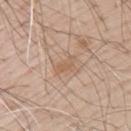Captured during whole-body skin photography for melanoma surveillance; the lesion was not biopsied.
A roughly 15 mm field-of-view crop from a total-body skin photograph.
Approximately 2.5 mm at its widest.
The lesion is located on the upper back.
The subject is a male aged 68–72.
This is a white-light tile.
Automated tile analysis of the lesion measured an area of roughly 2.5 mm² and a shape eccentricity near 0.9. The software also gave a classifier nevus-likeness of about 0/100.